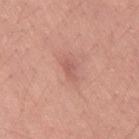biopsy status = no biopsy performed (imaged during a skin exam); subject = female, aged 23 to 27; body site = the left thigh; image = ~15 mm crop, total-body skin-cancer survey.